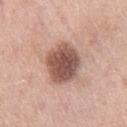Q: Was this lesion biopsied?
A: imaged on a skin check; not biopsied
Q: Illumination type?
A: white-light
Q: Where on the body is the lesion?
A: the right thigh
Q: Automated lesion metrics?
A: an average lesion color of about L≈53 a*≈21 b*≈25 (CIELAB), roughly 17 lightness units darker than nearby skin, and a lesion-to-skin contrast of about 11 (normalized; higher = more distinct); a border-irregularity rating of about 1.5/10, a within-lesion color-variation index near 5/10, and radial color variation of about 1.5
Q: What is the imaging modality?
A: total-body-photography crop, ~15 mm field of view
Q: Lesion size?
A: ~5 mm (longest diameter)
Q: What are the patient's age and sex?
A: female, aged 53 to 57